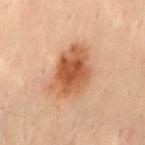Imaged during a routine full-body skin examination; the lesion was not biopsied and no histopathology is available.
The lesion is located on the mid back.
A 15 mm crop from a total-body photograph taken for skin-cancer surveillance.
The total-body-photography lesion software estimated a lesion area of about 19 mm² and an eccentricity of roughly 0.75. And it measured an automated nevus-likeness rating near 100 out of 100 and lesion-presence confidence of about 100/100.
This is a cross-polarized tile.
A male patient about 30 years old.